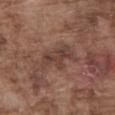Imaged during a routine full-body skin examination; the lesion was not biopsied and no histopathology is available. A male patient approximately 75 years of age. Longest diameter approximately 4.5 mm. Automated image analysis of the tile measured an area of roughly 8 mm², a shape eccentricity near 0.8, and two-axis asymmetry of about 0.45. It also reported a lesion color around L≈40 a*≈18 b*≈24 in CIELAB, a lesion–skin lightness drop of about 8, and a lesion-to-skin contrast of about 6.5 (normalized; higher = more distinct). Captured under white-light illumination. Cropped from a total-body skin-imaging series; the visible field is about 15 mm. The lesion is located on the front of the torso.